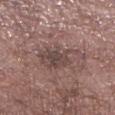Part of a total-body skin-imaging series; this lesion was reviewed on a skin check and was not flagged for biopsy.
An algorithmic analysis of the crop reported a mean CIELAB color near L≈45 a*≈17 b*≈19, a lesion–skin lightness drop of about 8, and a lesion-to-skin contrast of about 7 (normalized; higher = more distinct). And it measured border irregularity of about 7.5 on a 0–10 scale, internal color variation of about 4 on a 0–10 scale, and a peripheral color-asymmetry measure near 1.5. And it measured a classifier nevus-likeness of about 0/100 and a detector confidence of about 100 out of 100 that the crop contains a lesion.
From the right lower leg.
The subject is a male aged approximately 55.
This image is a 15 mm lesion crop taken from a total-body photograph.
Imaged with white-light lighting.
The recorded lesion diameter is about 5.5 mm.